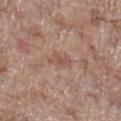The lesion was tiled from a total-body skin photograph and was not biopsied.
Cropped from a whole-body photographic skin survey; the tile spans about 15 mm.
Automated tile analysis of the lesion measured an eccentricity of roughly 0.9. And it measured a classifier nevus-likeness of about 0/100.
The lesion is on the right lower leg.
About 3 mm across.
Imaged with white-light lighting.
A male patient aged around 70.